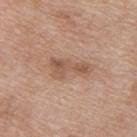Impression:
Recorded during total-body skin imaging; not selected for excision or biopsy.
Clinical summary:
The lesion is on the upper back. This is a white-light tile. A close-up tile cropped from a whole-body skin photograph, about 15 mm across. The patient is a female aged 38–42. An algorithmic analysis of the crop reported a footprint of about 8 mm², a shape eccentricity near 0.9, and a shape-asymmetry score of about 0.45 (0 = symmetric). The analysis additionally found a lesion color around L≈55 a*≈19 b*≈29 in CIELAB and a normalized border contrast of about 6.5.